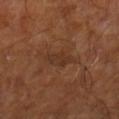Findings:
• follow-up · imaged on a skin check; not biopsied
• image-analysis metrics · a footprint of about 5 mm² and a shape eccentricity near 0.9; a classifier nevus-likeness of about 0/100 and a lesion-detection confidence of about 80/100
• anatomic site · the right lower leg
• illumination · cross-polarized illumination
• diameter · ~4 mm (longest diameter)
• image · ~15 mm crop, total-body skin-cancer survey
• patient · male, aged 58–62